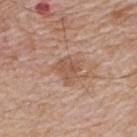<case>
<biopsy_status>not biopsied; imaged during a skin examination</biopsy_status>
<lesion_size>
  <long_diameter_mm_approx>2.5</long_diameter_mm_approx>
</lesion_size>
<image>
  <source>total-body photography crop</source>
  <field_of_view_mm>15</field_of_view_mm>
</image>
<lighting>white-light</lighting>
<site>back</site>
<patient>
  <sex>male</sex>
  <age_approx>65</age_approx>
</patient>
</case>